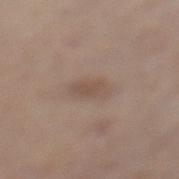workup: catalogued during a skin exam; not biopsied | anatomic site: the left lower leg | image: ~15 mm crop, total-body skin-cancer survey | subject: male, aged 63–67.